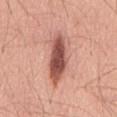biopsy status: catalogued during a skin exam; not biopsied
tile lighting: white-light
subject: male, aged 58–62
lesion diameter: ≈7.5 mm
image: total-body-photography crop, ~15 mm field of view
anatomic site: the mid back